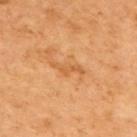The lesion was tiled from a total-body skin photograph and was not biopsied. The subject is a male in their mid- to late 60s. The recorded lesion diameter is about 4 mm. On the back. An algorithmic analysis of the crop reported border irregularity of about 7 on a 0–10 scale, internal color variation of about 0 on a 0–10 scale, and peripheral color asymmetry of about 0. The analysis additionally found a nevus-likeness score of about 0/100 and lesion-presence confidence of about 100/100. The tile uses cross-polarized illumination. A 15 mm close-up tile from a total-body photography series done for melanoma screening.